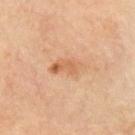follow-up: no biopsy performed (imaged during a skin exam)
lighting: cross-polarized
size: about 4 mm
image source: 15 mm crop, total-body photography
image-analysis metrics: a lesion color around L≈61 a*≈22 b*≈36 in CIELAB, about 8 CIELAB-L* units darker than the surrounding skin, and a normalized lesion–skin contrast near 6; a border-irregularity rating of about 3.5/10 and a color-variation rating of about 5.5/10
subject: male, aged 63–67
site: the right upper arm